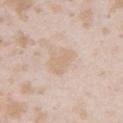Notes:
– acquisition — ~15 mm crop, total-body skin-cancer survey
– diameter — ≈3.5 mm
– illumination — white-light
– anatomic site — the left upper arm
– subject — female, aged 23 to 27